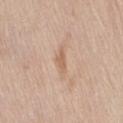notes: imaged on a skin check; not biopsied
imaging modality: ~15 mm tile from a whole-body skin photo
diameter: ~3 mm (longest diameter)
site: the lower back
lighting: white-light
patient: female, about 65 years old